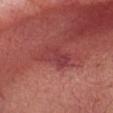No biopsy was performed on this lesion — it was imaged during a full skin examination and was not determined to be concerning. Cropped from a total-body skin-imaging series; the visible field is about 15 mm. The patient is a male about 65 years old. The lesion is on the head or neck.